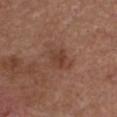| feature | finding |
|---|---|
| follow-up | catalogued during a skin exam; not biopsied |